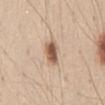Acquisition and patient details:
A male patient, aged around 45. The lesion is on the abdomen. A close-up tile cropped from a whole-body skin photograph, about 15 mm across.